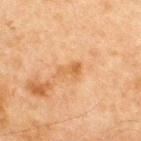  biopsy_status: not biopsied; imaged during a skin examination
  lesion_size:
    long_diameter_mm_approx: 2.5
  site: chest
  lighting: cross-polarized
  image:
    source: total-body photography crop
    field_of_view_mm: 15
  patient:
    sex: male
    age_approx: 65